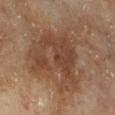The lesion was tiled from a total-body skin photograph and was not biopsied. Automated tile analysis of the lesion measured a footprint of about 49 mm² and a shape-asymmetry score of about 0.4 (0 = symmetric). It also reported a mean CIELAB color near L≈45 a*≈19 b*≈29. And it measured a border-irregularity rating of about 6.5/10, a color-variation rating of about 5.5/10, and peripheral color asymmetry of about 2. It also reported a nevus-likeness score of about 25/100 and a detector confidence of about 100 out of 100 that the crop contains a lesion. A male patient, aged around 65. Captured under cross-polarized illumination. Cropped from a whole-body photographic skin survey; the tile spans about 15 mm. From the right lower leg.